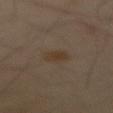follow-up: catalogued during a skin exam; not biopsied | patient: male, approximately 65 years of age | body site: the abdomen | image-analysis metrics: an area of roughly 4 mm², an outline eccentricity of about 0.75 (0 = round, 1 = elongated), and a symmetry-axis asymmetry near 0.2; a border-irregularity rating of about 2/10 and a peripheral color-asymmetry measure near 0.5 | tile lighting: cross-polarized illumination | acquisition: total-body-photography crop, ~15 mm field of view | diameter: about 2.5 mm.